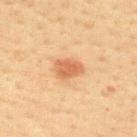Clinical impression: Recorded during total-body skin imaging; not selected for excision or biopsy. Background: Automated image analysis of the tile measured a mean CIELAB color near L≈54 a*≈23 b*≈35, a lesion–skin lightness drop of about 10, and a lesion-to-skin contrast of about 7.5 (normalized; higher = more distinct). The software also gave a nevus-likeness score of about 100/100 and a detector confidence of about 100 out of 100 that the crop contains a lesion. Captured under cross-polarized illumination. On the upper back. The lesion's longest dimension is about 3.5 mm. A region of skin cropped from a whole-body photographic capture, roughly 15 mm wide. A female patient, aged approximately 40.